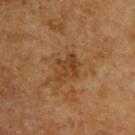  biopsy_status: not biopsied; imaged during a skin examination
  automated_metrics:
    area_mm2_approx: 6.5
    eccentricity: 0.65
    shape_asymmetry: 0.35
    border_irregularity_0_10: 4.5
    color_variation_0_10: 3.0
    peripheral_color_asymmetry: 1.0
    nevus_likeness_0_100: 0
    lesion_detection_confidence_0_100: 100
  site: upper back
  lesion_size:
    long_diameter_mm_approx: 3.5
  patient:
    sex: male
    age_approx: 65
  image:
    source: total-body photography crop
    field_of_view_mm: 15